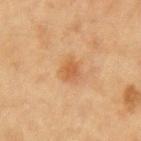A female subject roughly 40 years of age.
Located on the left upper arm.
This is a cross-polarized tile.
A 15 mm crop from a total-body photograph taken for skin-cancer surveillance.